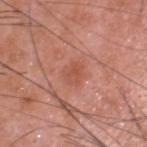follow-up: total-body-photography surveillance lesion; no biopsy
subject: male, aged 58–62
lesion size: ~2.5 mm (longest diameter)
anatomic site: the head or neck
tile lighting: white-light illumination
image source: ~15 mm tile from a whole-body skin photo
image-analysis metrics: a lesion area of about 5 mm², an eccentricity of roughly 0.25, and a symmetry-axis asymmetry near 0.15; a mean CIELAB color near L≈53 a*≈27 b*≈31, a lesion–skin lightness drop of about 7, and a normalized border contrast of about 5.5; a border-irregularity rating of about 1.5/10, a color-variation rating of about 2.5/10, and radial color variation of about 1; a nevus-likeness score of about 0/100